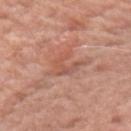– tile lighting — white-light illumination
– location — the arm
– lesion size — ≈3 mm
– image source — ~15 mm crop, total-body skin-cancer survey
– image-analysis metrics — a footprint of about 5 mm²
– subject — male, in their mid-60s